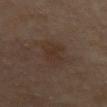This lesion was catalogued during total-body skin photography and was not selected for biopsy.
From the abdomen.
A close-up tile cropped from a whole-body skin photograph, about 15 mm across.
A female subject, aged around 50.
Captured under cross-polarized illumination.